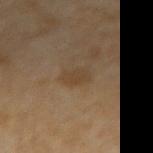Findings:
• workup: total-body-photography surveillance lesion; no biopsy
• image source: total-body-photography crop, ~15 mm field of view
• patient: female, in their 60s
• site: the left forearm